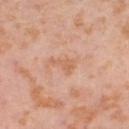Clinical impression:
The lesion was tiled from a total-body skin photograph and was not biopsied.
Context:
The recorded lesion diameter is about 3 mm. A 15 mm close-up tile from a total-body photography series done for melanoma screening. The tile uses cross-polarized illumination. The patient is a female aged 53 to 57. The total-body-photography lesion software estimated internal color variation of about 1 on a 0–10 scale and radial color variation of about 0.5. On the right thigh.